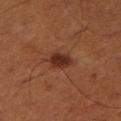| field | value |
|---|---|
| biopsy status | imaged on a skin check; not biopsied |
| subject | male, roughly 50 years of age |
| acquisition | total-body-photography crop, ~15 mm field of view |
| lighting | cross-polarized illumination |
| lesion size | ≈3 mm |
| automated lesion analysis | a mean CIELAB color near L≈30 a*≈24 b*≈29, roughly 11 lightness units darker than nearby skin, and a normalized border contrast of about 10; lesion-presence confidence of about 100/100 |
| location | the right thigh |